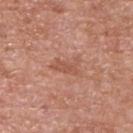Assessment: This lesion was catalogued during total-body skin photography and was not selected for biopsy.